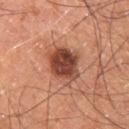Part of a total-body skin-imaging series; this lesion was reviewed on a skin check and was not flagged for biopsy.
From the right thigh.
A 15 mm crop from a total-body photograph taken for skin-cancer surveillance.
A male subject in their 60s.
Imaged with cross-polarized lighting.
The recorded lesion diameter is about 4.5 mm.